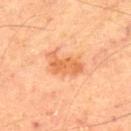Recorded during total-body skin imaging; not selected for excision or biopsy. Captured under cross-polarized illumination. Cropped from a whole-body photographic skin survey; the tile spans about 15 mm. Located on the upper back. A male subject, aged approximately 65.